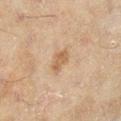Recorded during total-body skin imaging; not selected for excision or biopsy.
The patient is a female about 60 years old.
The tile uses cross-polarized illumination.
About 2.5 mm across.
The lesion is on the right lower leg.
A 15 mm close-up tile from a total-body photography series done for melanoma screening.